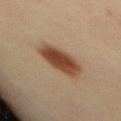- lesion size · ~4.5 mm (longest diameter)
- subject · female, about 35 years old
- image · ~15 mm tile from a whole-body skin photo
- body site · the mid back
- tile lighting · cross-polarized